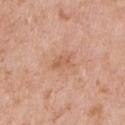This lesion was catalogued during total-body skin photography and was not selected for biopsy. The recorded lesion diameter is about 2.5 mm. This is a white-light tile. The lesion-visualizer software estimated an area of roughly 3.5 mm², an outline eccentricity of about 0.85 (0 = round, 1 = elongated), and a shape-asymmetry score of about 0.3 (0 = symmetric). The analysis additionally found a lesion color around L≈60 a*≈23 b*≈34 in CIELAB, roughly 7 lightness units darker than nearby skin, and a lesion-to-skin contrast of about 5.5 (normalized; higher = more distinct). It also reported a border-irregularity rating of about 3/10 and radial color variation of about 0.5. Cropped from a whole-body photographic skin survey; the tile spans about 15 mm. A female subject, aged 38 to 42. The lesion is located on the front of the torso.